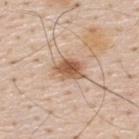Captured during whole-body skin photography for melanoma surveillance; the lesion was not biopsied. The lesion's longest dimension is about 4 mm. The lesion is on the back. The subject is a male aged 58–62. The lesion-visualizer software estimated a lesion area of about 8 mm² and an eccentricity of roughly 0.75. The analysis additionally found a within-lesion color-variation index near 4.5/10 and radial color variation of about 1.5. A 15 mm close-up extracted from a 3D total-body photography capture.